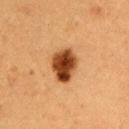Assessment:
This lesion was catalogued during total-body skin photography and was not selected for biopsy.
Image and clinical context:
A region of skin cropped from a whole-body photographic capture, roughly 15 mm wide. On the left upper arm. Approximately 4 mm at its widest. The subject is a male about 50 years old.